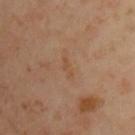The subject is a male roughly 45 years of age. Automated tile analysis of the lesion measured an area of roughly 2 mm², an outline eccentricity of about 0.95 (0 = round, 1 = elongated), and a shape-asymmetry score of about 0.45 (0 = symmetric). It also reported a lesion color around L≈48 a*≈19 b*≈33 in CIELAB and a lesion–skin lightness drop of about 5. A region of skin cropped from a whole-body photographic capture, roughly 15 mm wide. Approximately 2.5 mm at its widest. This is a cross-polarized tile. The lesion is on the front of the torso.